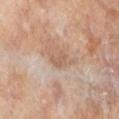Q: Was this lesion biopsied?
A: no biopsy performed (imaged during a skin exam)
Q: What is the imaging modality?
A: ~15 mm tile from a whole-body skin photo
Q: Where on the body is the lesion?
A: the right lower leg
Q: Patient demographics?
A: female, about 75 years old
Q: What is the lesion's diameter?
A: ~2.5 mm (longest diameter)
Q: How was the tile lit?
A: cross-polarized